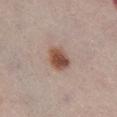- notes — total-body-photography surveillance lesion; no biopsy
- acquisition — total-body-photography crop, ~15 mm field of view
- lesion diameter — ~3.5 mm (longest diameter)
- location — the chest
- subject — female, aged 63–67